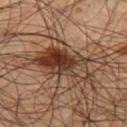The lesion-visualizer software estimated an area of roughly 17 mm², a shape eccentricity near 0.9, and a symmetry-axis asymmetry near 0.3. The analysis additionally found a lesion color around L≈36 a*≈19 b*≈27 in CIELAB, a lesion–skin lightness drop of about 13, and a lesion-to-skin contrast of about 11 (normalized; higher = more distinct). The software also gave a within-lesion color-variation index near 10/10 and radial color variation of about 3.5. And it measured a detector confidence of about 100 out of 100 that the crop contains a lesion.
Approximately 7.5 mm at its widest.
This is a cross-polarized tile.
A male subject aged around 45.
The lesion is located on the left thigh.
A 15 mm crop from a total-body photograph taken for skin-cancer surveillance.